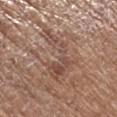Impression:
Part of a total-body skin-imaging series; this lesion was reviewed on a skin check and was not flagged for biopsy.
Context:
A close-up tile cropped from a whole-body skin photograph, about 15 mm across. Imaged with white-light lighting. The lesion's longest dimension is about 7.5 mm. The lesion is on the leg. A female patient, about 75 years old.